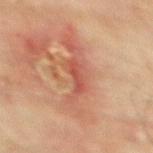Assessment: The lesion was photographed on a routine skin check and not biopsied; there is no pathology result. Context: Imaged with cross-polarized lighting. The lesion's longest dimension is about 3.5 mm. The patient is a male aged around 75. The lesion is located on the upper back. A 15 mm crop from a total-body photograph taken for skin-cancer surveillance.